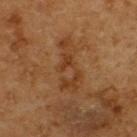Part of a total-body skin-imaging series; this lesion was reviewed on a skin check and was not flagged for biopsy. About 6.5 mm across. The lesion-visualizer software estimated a lesion area of about 12 mm², an outline eccentricity of about 0.95 (0 = round, 1 = elongated), and a shape-asymmetry score of about 0.4 (0 = symmetric). It also reported a mean CIELAB color near L≈36 a*≈20 b*≈32, about 7 CIELAB-L* units darker than the surrounding skin, and a normalized lesion–skin contrast near 6. It also reported a classifier nevus-likeness of about 0/100 and lesion-presence confidence of about 80/100. The tile uses cross-polarized illumination. The patient is a male aged around 60. The lesion is on the back. Cropped from a total-body skin-imaging series; the visible field is about 15 mm.